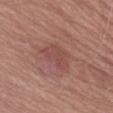workup = no biopsy performed (imaged during a skin exam) | tile lighting = white-light illumination | anatomic site = the left thigh | patient = female, aged approximately 70 | lesion size = ≈4.5 mm | imaging modality = ~15 mm tile from a whole-body skin photo.